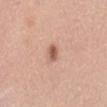Impression: Imaged during a routine full-body skin examination; the lesion was not biopsied and no histopathology is available. Image and clinical context: Longest diameter approximately 2.5 mm. Located on the mid back. A female subject, approximately 65 years of age. Imaged with white-light lighting. The lesion-visualizer software estimated a footprint of about 3 mm², an outline eccentricity of about 0.8 (0 = round, 1 = elongated), and a symmetry-axis asymmetry near 0.3. The analysis additionally found a within-lesion color-variation index near 1.5/10 and radial color variation of about 0.5. This image is a 15 mm lesion crop taken from a total-body photograph.